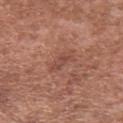Imaged during a routine full-body skin examination; the lesion was not biopsied and no histopathology is available. A male subject aged approximately 45. Cropped from a whole-body photographic skin survey; the tile spans about 15 mm. The lesion is located on the upper back.